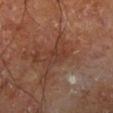Q: Was a biopsy performed?
A: total-body-photography surveillance lesion; no biopsy
Q: Patient demographics?
A: male, aged around 60
Q: What did automated image analysis measure?
A: about 6 CIELAB-L* units darker than the surrounding skin and a normalized border contrast of about 5.5; an automated nevus-likeness rating near 0 out of 100 and a lesion-detection confidence of about 90/100
Q: What is the imaging modality?
A: 15 mm crop, total-body photography
Q: What lighting was used for the tile?
A: cross-polarized illumination
Q: Where on the body is the lesion?
A: the right leg